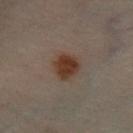Assessment:
The lesion was photographed on a routine skin check and not biopsied; there is no pathology result.
Background:
This image is a 15 mm lesion crop taken from a total-body photograph. A male patient aged 48–52. The lesion is on the left leg. Imaged with cross-polarized lighting. Longest diameter approximately 3.5 mm.